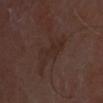Clinical impression: Recorded during total-body skin imaging; not selected for excision or biopsy. Clinical summary: Approximately 5 mm at its widest. The patient is a male roughly 65 years of age. The lesion is located on the head or neck. A 15 mm close-up extracted from a 3D total-body photography capture.